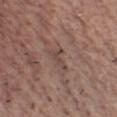Q: Is there a histopathology result?
A: catalogued during a skin exam; not biopsied
Q: What lighting was used for the tile?
A: white-light
Q: Who is the patient?
A: male, in their mid-50s
Q: Lesion location?
A: the chest
Q: Lesion size?
A: about 3 mm
Q: How was this image acquired?
A: ~15 mm tile from a whole-body skin photo